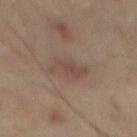{
  "biopsy_status": "not biopsied; imaged during a skin examination",
  "patient": {
    "sex": "male",
    "age_approx": 65
  },
  "site": "mid back",
  "image": {
    "source": "total-body photography crop",
    "field_of_view_mm": 15
  }
}